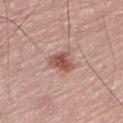No biopsy was performed on this lesion — it was imaged during a full skin examination and was not determined to be concerning. The patient is a male aged 73–77. Imaged with white-light lighting. Longest diameter approximately 3.5 mm. Located on the back. A region of skin cropped from a whole-body photographic capture, roughly 15 mm wide.